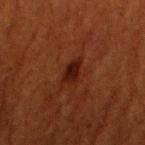Findings:
* biopsy status: imaged on a skin check; not biopsied
* subject: female, approximately 80 years of age
* diameter: ~3 mm (longest diameter)
* illumination: cross-polarized
* site: the left upper arm
* imaging modality: ~15 mm tile from a whole-body skin photo